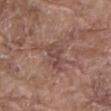patient: male, approximately 80 years of age | lighting: white-light | automated lesion analysis: a lesion area of about 7 mm², a shape eccentricity near 0.75, and two-axis asymmetry of about 0.35; a lesion color around L≈46 a*≈19 b*≈22 in CIELAB, a lesion–skin lightness drop of about 7, and a lesion-to-skin contrast of about 6 (normalized; higher = more distinct); internal color variation of about 3.5 on a 0–10 scale and a peripheral color-asymmetry measure near 1.5; a classifier nevus-likeness of about 0/100 | image: ~15 mm crop, total-body skin-cancer survey | anatomic site: the mid back | lesion size: about 4 mm.